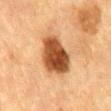follow-up: catalogued during a skin exam; not biopsied
location: the mid back
patient: male, in their mid-80s or older
image source: ~15 mm tile from a whole-body skin photo